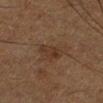Case summary:
– biopsy status — catalogued during a skin exam; not biopsied
– tile lighting — cross-polarized illumination
– patient — male, in their mid-50s
– automated lesion analysis — a lesion color around L≈29 a*≈15 b*≈24 in CIELAB and a normalized border contrast of about 6; a border-irregularity rating of about 2/10 and radial color variation of about 1; a classifier nevus-likeness of about 0/100 and lesion-presence confidence of about 100/100
– acquisition — 15 mm crop, total-body photography
– anatomic site — the left lower leg
– lesion size — ~2.5 mm (longest diameter)